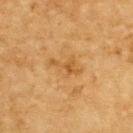Impression: Recorded during total-body skin imaging; not selected for excision or biopsy. Image and clinical context: About 3.5 mm across. A roughly 15 mm field-of-view crop from a total-body skin photograph. This is a cross-polarized tile. The lesion is on the upper back. A male patient approximately 85 years of age or older.